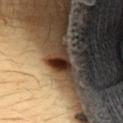| key | value |
|---|---|
| biopsy status | catalogued during a skin exam; not biopsied |
| imaging modality | ~15 mm crop, total-body skin-cancer survey |
| anatomic site | the upper back |
| lesion size | ≈3.5 mm |
| illumination | cross-polarized illumination |
| image-analysis metrics | a lesion–skin lightness drop of about 17 and a normalized lesion–skin contrast near 14.5; a detector confidence of about 95 out of 100 that the crop contains a lesion |
| subject | female, roughly 35 years of age |